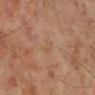Q: How was the tile lit?
A: cross-polarized illumination
Q: Lesion location?
A: the right lower leg
Q: What is the imaging modality?
A: 15 mm crop, total-body photography
Q: Lesion size?
A: about 2 mm
Q: Who is the patient?
A: male, approximately 70 years of age